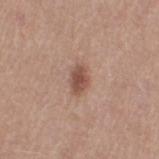follow-up: total-body-photography surveillance lesion; no biopsy | imaging modality: total-body-photography crop, ~15 mm field of view | TBP lesion metrics: a lesion area of about 4.5 mm², an outline eccentricity of about 0.8 (0 = round, 1 = elongated), and a symmetry-axis asymmetry near 0.2; an average lesion color of about L≈50 a*≈21 b*≈26 (CIELAB), roughly 12 lightness units darker than nearby skin, and a normalized lesion–skin contrast near 8.5; a nevus-likeness score of about 90/100 and lesion-presence confidence of about 100/100 | subject: female, aged around 55 | site: the left thigh | illumination: white-light | lesion size: ~3 mm (longest diameter).